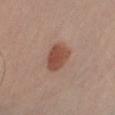Recorded during total-body skin imaging; not selected for excision or biopsy. The total-body-photography lesion software estimated an average lesion color of about L≈48 a*≈23 b*≈28 (CIELAB) and a normalized lesion–skin contrast near 9. The software also gave a border-irregularity index near 2/10, internal color variation of about 2.5 on a 0–10 scale, and radial color variation of about 0.5. A region of skin cropped from a whole-body photographic capture, roughly 15 mm wide. Longest diameter approximately 3.5 mm. The lesion is located on the right upper arm. The patient is a male about 55 years old.